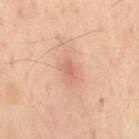subject = male, aged approximately 55
anatomic site = the mid back
image = total-body-photography crop, ~15 mm field of view